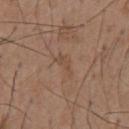This lesion was catalogued during total-body skin photography and was not selected for biopsy.